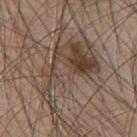{
  "patient": {
    "sex": "male",
    "age_approx": 45
  },
  "lighting": "white-light",
  "automated_metrics": {
    "border_irregularity_0_10": 10.0,
    "nevus_likeness_0_100": 30
  },
  "lesion_size": {
    "long_diameter_mm_approx": 10.5
  },
  "image": {
    "source": "total-body photography crop",
    "field_of_view_mm": 15
  },
  "site": "mid back"
}